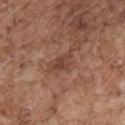biopsy status: total-body-photography surveillance lesion; no biopsy
lesion size: about 3 mm
acquisition: ~15 mm crop, total-body skin-cancer survey
illumination: white-light
site: the chest
patient: male, aged approximately 70